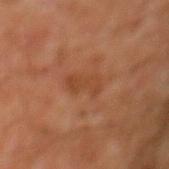follow-up — imaged on a skin check; not biopsied | patient — male, in their 60s | location — the chest | illumination — cross-polarized illumination | lesion size — ~3.5 mm (longest diameter) | imaging modality — ~15 mm tile from a whole-body skin photo | image-analysis metrics — a color-variation rating of about 2/10.